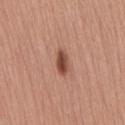This lesion was catalogued during total-body skin photography and was not selected for biopsy.
Imaged with white-light lighting.
On the lower back.
About 3 mm across.
The patient is a female approximately 45 years of age.
Cropped from a total-body skin-imaging series; the visible field is about 15 mm.
Automated tile analysis of the lesion measured an outline eccentricity of about 0.85 (0 = round, 1 = elongated). It also reported an average lesion color of about L≈48 a*≈25 b*≈29 (CIELAB), roughly 14 lightness units darker than nearby skin, and a lesion-to-skin contrast of about 10 (normalized; higher = more distinct).